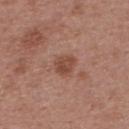Findings:
* diameter — ≈2.5 mm
* illumination — white-light illumination
* acquisition — ~15 mm tile from a whole-body skin photo
* anatomic site — the upper back
* subject — female, aged approximately 50
* automated lesion analysis — a normalized lesion–skin contrast near 7; a border-irregularity rating of about 2.5/10 and a color-variation rating of about 2.5/10; an automated nevus-likeness rating near 40 out of 100